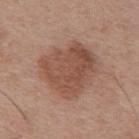Recorded during total-body skin imaging; not selected for excision or biopsy.
A male subject roughly 55 years of age.
On the mid back.
A 15 mm close-up tile from a total-body photography series done for melanoma screening.